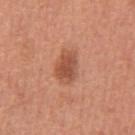Assessment: No biopsy was performed on this lesion — it was imaged during a full skin examination and was not determined to be concerning. Context: A close-up tile cropped from a whole-body skin photograph, about 15 mm across. On the abdomen. A male patient approximately 65 years of age. The recorded lesion diameter is about 4 mm. Automated tile analysis of the lesion measured an area of roughly 8 mm², an outline eccentricity of about 0.75 (0 = round, 1 = elongated), and a symmetry-axis asymmetry near 0.2. It also reported a mean CIELAB color near L≈52 a*≈26 b*≈32, about 11 CIELAB-L* units darker than the surrounding skin, and a lesion-to-skin contrast of about 7.5 (normalized; higher = more distinct). The analysis additionally found a border-irregularity rating of about 2/10, a color-variation rating of about 3/10, and a peripheral color-asymmetry measure near 1. Captured under white-light illumination.